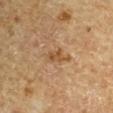The lesion was tiled from a total-body skin photograph and was not biopsied. The lesion is on the left upper arm. The recorded lesion diameter is about 3.5 mm. A region of skin cropped from a whole-body photographic capture, roughly 15 mm wide. This is a cross-polarized tile. A male patient, aged around 65.